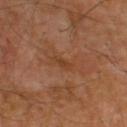biopsy_status: not biopsied; imaged during a skin examination
patient:
  sex: male
  age_approx: 55
image:
  source: total-body photography crop
  field_of_view_mm: 15
site: upper back